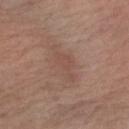Recorded during total-body skin imaging; not selected for excision or biopsy.